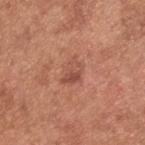{"biopsy_status": "not biopsied; imaged during a skin examination", "patient": {"sex": "male", "age_approx": 55}, "automated_metrics": {"cielab_L": 51, "cielab_a": 25, "cielab_b": 30, "vs_skin_darker_L": 8.0, "vs_skin_contrast_norm": 5.5, "peripheral_color_asymmetry": 2.5}, "site": "back", "image": {"source": "total-body photography crop", "field_of_view_mm": 15}}